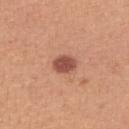Impression: This lesion was catalogued during total-body skin photography and was not selected for biopsy. Clinical summary: The lesion is on the right upper arm. Cropped from a total-body skin-imaging series; the visible field is about 15 mm. A female patient, about 45 years old.